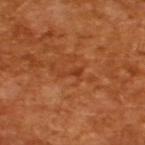Clinical summary:
A roughly 15 mm field-of-view crop from a total-body skin photograph. Approximately 2.5 mm at its widest. This is a cross-polarized tile. A male subject in their mid- to late 60s.